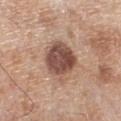Recorded during total-body skin imaging; not selected for excision or biopsy. The lesion is on the left lower leg. The subject is a male aged around 80. The tile uses white-light illumination. Cropped from a whole-body photographic skin survey; the tile spans about 15 mm. Measured at roughly 4.5 mm in maximum diameter.